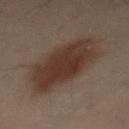{
  "biopsy_status": "not biopsied; imaged during a skin examination",
  "site": "lower back",
  "patient": {
    "sex": "male",
    "age_approx": 50
  },
  "automated_metrics": {
    "area_mm2_approx": 31.0,
    "eccentricity": 0.85,
    "shape_asymmetry": 0.1,
    "cielab_L": 27,
    "cielab_a": 13,
    "cielab_b": 19,
    "vs_skin_darker_L": 9.0,
    "vs_skin_contrast_norm": 10.0
  },
  "image": {
    "source": "total-body photography crop",
    "field_of_view_mm": 15
  }
}